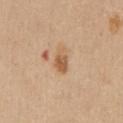No biopsy was performed on this lesion — it was imaged during a full skin examination and was not determined to be concerning. The patient is a male aged 38–42. The lesion's longest dimension is about 3 mm. Located on the chest. Cropped from a whole-body photographic skin survey; the tile spans about 15 mm.